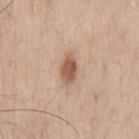<lesion>
  <biopsy_status>not biopsied; imaged during a skin examination</biopsy_status>
  <site>chest</site>
  <automated_metrics>
    <area_mm2_approx>6.0</area_mm2_approx>
    <eccentricity>0.85</eccentricity>
    <shape_asymmetry>0.25</shape_asymmetry>
  </automated_metrics>
  <image>
    <source>total-body photography crop</source>
    <field_of_view_mm>15</field_of_view_mm>
  </image>
  <patient>
    <sex>male</sex>
    <age_approx>70</age_approx>
  </patient>
  <lesion_size>
    <long_diameter_mm_approx>3.5</long_diameter_mm_approx>
  </lesion_size>
</lesion>